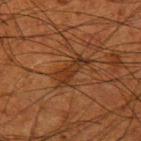workup: total-body-photography surveillance lesion; no biopsy | site: the left upper arm | imaging modality: total-body-photography crop, ~15 mm field of view | patient: male, about 50 years old.